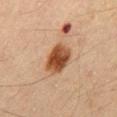Clinical impression: Captured during whole-body skin photography for melanoma surveillance; the lesion was not biopsied. Acquisition and patient details: A male patient aged 63 to 67. From the mid back. Cropped from a total-body skin-imaging series; the visible field is about 15 mm.